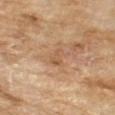This image is a 15 mm lesion crop taken from a total-body photograph. A male subject in their mid- to late 60s. The tile uses cross-polarized illumination.